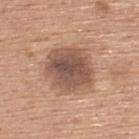| key | value |
|---|---|
| follow-up | no biopsy performed (imaged during a skin exam) |
| image | 15 mm crop, total-body photography |
| subject | female, roughly 60 years of age |
| location | the upper back |